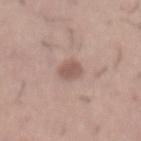Assessment:
Part of a total-body skin-imaging series; this lesion was reviewed on a skin check and was not flagged for biopsy.
Background:
On the abdomen. This is a white-light tile. A male patient in their mid- to late 40s. The total-body-photography lesion software estimated a footprint of about 4 mm² and an eccentricity of roughly 0.7. A roughly 15 mm field-of-view crop from a total-body skin photograph. About 2.5 mm across.